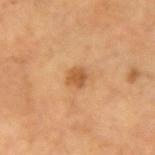Imaged during a routine full-body skin examination; the lesion was not biopsied and no histopathology is available.
The lesion is located on the right forearm.
A lesion tile, about 15 mm wide, cut from a 3D total-body photograph.
Longest diameter approximately 2 mm.
The subject is a female aged around 70.